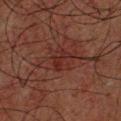Q: Was this lesion biopsied?
A: imaged on a skin check; not biopsied
Q: How was the tile lit?
A: cross-polarized illumination
Q: What is the anatomic site?
A: the chest
Q: Who is the patient?
A: male, aged 58 to 62
Q: How was this image acquired?
A: ~15 mm tile from a whole-body skin photo
Q: How large is the lesion?
A: ≈5.5 mm